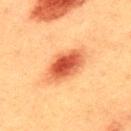Imaged during a routine full-body skin examination; the lesion was not biopsied and no histopathology is available. A 15 mm close-up tile from a total-body photography series done for melanoma screening. A male patient, roughly 40 years of age. An algorithmic analysis of the crop reported an area of roughly 12 mm², an outline eccentricity of about 0.85 (0 = round, 1 = elongated), and a shape-asymmetry score of about 0.15 (0 = symmetric). And it measured a mean CIELAB color near L≈49 a*≈30 b*≈37, a lesion–skin lightness drop of about 15, and a normalized border contrast of about 10. The analysis additionally found a border-irregularity rating of about 2/10 and a within-lesion color-variation index near 6.5/10. It also reported a nevus-likeness score of about 100/100. Longest diameter approximately 5.5 mm. Located on the upper back. This is a cross-polarized tile.